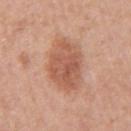• notes — catalogued during a skin exam; not biopsied
• subject — female, roughly 40 years of age
• site — the right upper arm
• automated lesion analysis — a footprint of about 19 mm², an outline eccentricity of about 0.65 (0 = round, 1 = elongated), and a shape-asymmetry score of about 0.1 (0 = symmetric); an automated nevus-likeness rating near 50 out of 100
• illumination — white-light
• image source — ~15 mm crop, total-body skin-cancer survey